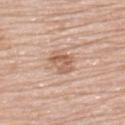Impression:
Captured during whole-body skin photography for melanoma surveillance; the lesion was not biopsied.
Context:
The lesion's longest dimension is about 3 mm. The tile uses white-light illumination. A female subject aged around 65. The lesion is on the upper back. A region of skin cropped from a whole-body photographic capture, roughly 15 mm wide.